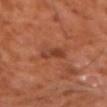Cropped from a whole-body photographic skin survey; the tile spans about 15 mm.
Captured under cross-polarized illumination.
From the left forearm.
A male subject in their 60s.